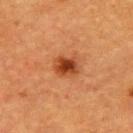  patient:
    sex: female
    age_approx: 55
  lighting: cross-polarized
  image:
    source: total-body photography crop
    field_of_view_mm: 15
  site: upper back